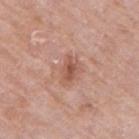subject: female, aged 68–72
image source: ~15 mm crop, total-body skin-cancer survey
illumination: white-light illumination
location: the right upper arm
lesion diameter: ~3 mm (longest diameter)